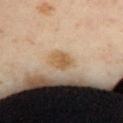workup: imaged on a skin check; not biopsied
lighting: cross-polarized illumination
automated metrics: a shape-asymmetry score of about 0.25 (0 = symmetric); a border-irregularity index near 2/10 and peripheral color asymmetry of about 1; a classifier nevus-likeness of about 35/100 and a lesion-detection confidence of about 100/100
image source: ~15 mm tile from a whole-body skin photo
diameter: ≈2.5 mm
patient: female, aged 38–42
anatomic site: the back